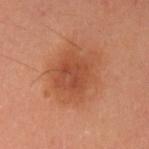Q: What is the lesion's diameter?
A: about 6 mm
Q: What is the anatomic site?
A: the left upper arm
Q: Automated lesion metrics?
A: a normalized border contrast of about 6.5; a border-irregularity index near 3.5/10
Q: Illumination type?
A: cross-polarized illumination
Q: What are the patient's age and sex?
A: female, in their mid- to late 30s
Q: How was this image acquired?
A: ~15 mm crop, total-body skin-cancer survey